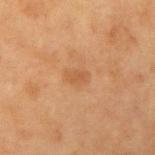Clinical impression:
Captured during whole-body skin photography for melanoma surveillance; the lesion was not biopsied.
Background:
Automated tile analysis of the lesion measured an eccentricity of roughly 0.75. It also reported an average lesion color of about L≈48 a*≈20 b*≈34 (CIELAB), a lesion–skin lightness drop of about 6, and a lesion-to-skin contrast of about 5 (normalized; higher = more distinct). It also reported border irregularity of about 2 on a 0–10 scale and internal color variation of about 1.5 on a 0–10 scale. This image is a 15 mm lesion crop taken from a total-body photograph. The lesion is on the right upper arm. A female subject aged around 40. This is a cross-polarized tile. Approximately 2.5 mm at its widest.